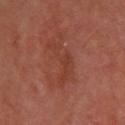The lesion was tiled from a total-body skin photograph and was not biopsied. The lesion is located on the upper back. A close-up tile cropped from a whole-body skin photograph, about 15 mm across. The patient is a female aged 53 to 57. Automated image analysis of the tile measured a footprint of about 8.5 mm², an eccentricity of roughly 0.9, and a shape-asymmetry score of about 0.7 (0 = symmetric). The analysis additionally found a lesion–skin lightness drop of about 6 and a normalized border contrast of about 5.5. And it measured an automated nevus-likeness rating near 0 out of 100 and a detector confidence of about 100 out of 100 that the crop contains a lesion.